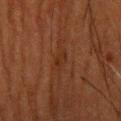Findings:
* notes: total-body-photography surveillance lesion; no biopsy
* location: the head or neck
* lesion diameter: about 3 mm
* patient: male, aged 48 to 52
* illumination: cross-polarized illumination
* image: ~15 mm tile from a whole-body skin photo
* automated metrics: an eccentricity of roughly 0.9 and a symmetry-axis asymmetry near 0.2; a mean CIELAB color near L≈23 a*≈18 b*≈25 and a lesion-to-skin contrast of about 5 (normalized; higher = more distinct); a border-irregularity index near 2.5/10 and a within-lesion color-variation index near 1.5/10; an automated nevus-likeness rating near 0 out of 100 and lesion-presence confidence of about 90/100